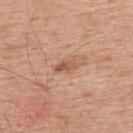Captured during whole-body skin photography for melanoma surveillance; the lesion was not biopsied.
The lesion is on the upper back.
The subject is a male approximately 55 years of age.
A 15 mm close-up extracted from a 3D total-body photography capture.
The lesion's longest dimension is about 2.5 mm.